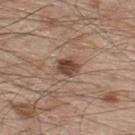Notes:
• follow-up · catalogued during a skin exam; not biopsied
• patient · male, about 50 years old
• body site · the upper back
• diameter · about 3 mm
• imaging modality · total-body-photography crop, ~15 mm field of view
• tile lighting · white-light illumination